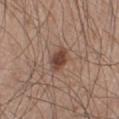Assessment:
The lesion was tiled from a total-body skin photograph and was not biopsied.
Clinical summary:
Measured at roughly 3 mm in maximum diameter. A male subject aged approximately 60. A close-up tile cropped from a whole-body skin photograph, about 15 mm across. Located on the right lower leg.